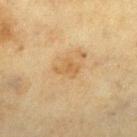Captured during whole-body skin photography for melanoma surveillance; the lesion was not biopsied. A female patient in their mid-50s. A close-up tile cropped from a whole-body skin photograph, about 15 mm across. The lesion is on the left lower leg.